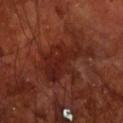Case summary:
• follow-up · catalogued during a skin exam; not biopsied
• anatomic site · the front of the torso
• image source · total-body-photography crop, ~15 mm field of view
• patient · male, aged around 70